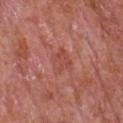Assessment:
No biopsy was performed on this lesion — it was imaged during a full skin examination and was not determined to be concerning.
Acquisition and patient details:
The recorded lesion diameter is about 3 mm. Automated tile analysis of the lesion measured a lesion area of about 6.5 mm², a shape eccentricity near 0.45, and a shape-asymmetry score of about 0.2 (0 = symmetric). The software also gave a lesion color around L≈49 a*≈29 b*≈29 in CIELAB, roughly 6 lightness units darker than nearby skin, and a lesion-to-skin contrast of about 4.5 (normalized; higher = more distinct). The software also gave border irregularity of about 2.5 on a 0–10 scale and a color-variation rating of about 2/10. The analysis additionally found a lesion-detection confidence of about 100/100. Located on the chest. A region of skin cropped from a whole-body photographic capture, roughly 15 mm wide. The patient is a male aged 63 to 67.